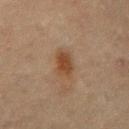Q: Was a biopsy performed?
A: catalogued during a skin exam; not biopsied
Q: What lighting was used for the tile?
A: cross-polarized illumination
Q: What is the anatomic site?
A: the mid back
Q: What did automated image analysis measure?
A: a mean CIELAB color near L≈37 a*≈16 b*≈28 and a normalized lesion–skin contrast near 8; a classifier nevus-likeness of about 85/100 and a lesion-detection confidence of about 100/100
Q: What is the imaging modality?
A: ~15 mm crop, total-body skin-cancer survey
Q: Who is the patient?
A: male, roughly 70 years of age
Q: How large is the lesion?
A: ≈3.5 mm